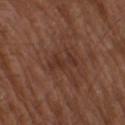Case summary:
– lighting — white-light
– site — the arm
– patient — male, about 75 years old
– image — total-body-photography crop, ~15 mm field of view
– size — ~3.5 mm (longest diameter)